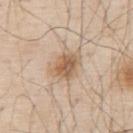The lesion was tiled from a total-body skin photograph and was not biopsied.
From the upper back.
An algorithmic analysis of the crop reported a shape-asymmetry score of about 0.15 (0 = symmetric). The analysis additionally found lesion-presence confidence of about 100/100.
The patient is a male roughly 80 years of age.
The tile uses white-light illumination.
Cropped from a whole-body photographic skin survey; the tile spans about 15 mm.